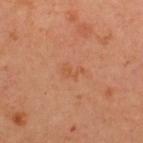follow-up=catalogued during a skin exam; not biopsied
body site=the upper back
image=total-body-photography crop, ~15 mm field of view
subject=female, in their mid-40s